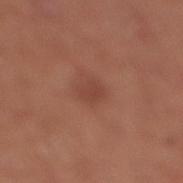  biopsy_status: not biopsied; imaged during a skin examination
  patient:
    sex: male
    age_approx: 70
  image:
    source: total-body photography crop
    field_of_view_mm: 15
  site: right lower leg
  automated_metrics:
    border_irregularity_0_10: 2.0
    color_variation_0_10: 1.0
    peripheral_color_asymmetry: 0.5
    nevus_likeness_0_100: 0
    lesion_detection_confidence_0_100: 100